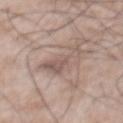<tbp_lesion>
<site>back</site>
<patient>
  <sex>male</sex>
  <age_approx>50</age_approx>
</patient>
<lighting>white-light</lighting>
<lesion_size>
  <long_diameter_mm_approx>5.5</long_diameter_mm_approx>
</lesion_size>
<image>
  <source>total-body photography crop</source>
  <field_of_view_mm>15</field_of_view_mm>
</image>
</tbp_lesion>